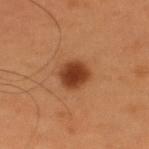Assessment:
Imaged during a routine full-body skin examination; the lesion was not biopsied and no histopathology is available.
Acquisition and patient details:
An algorithmic analysis of the crop reported an area of roughly 8.5 mm² and a symmetry-axis asymmetry near 0.15. A region of skin cropped from a whole-body photographic capture, roughly 15 mm wide. The lesion is located on the upper back. A male subject approximately 55 years of age.